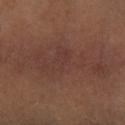Captured during whole-body skin photography for melanoma surveillance; the lesion was not biopsied.
The subject is a female aged 53–57.
This is a cross-polarized tile.
Automated image analysis of the tile measured an area of roughly 34 mm², an eccentricity of roughly 0.95, and a shape-asymmetry score of about 0.35 (0 = symmetric). It also reported border irregularity of about 6.5 on a 0–10 scale and a within-lesion color-variation index near 3/10.
Located on the left lower leg.
This image is a 15 mm lesion crop taken from a total-body photograph.
The lesion's longest dimension is about 12 mm.